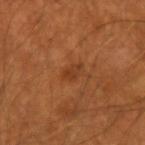Q: Was this lesion biopsied?
A: total-body-photography surveillance lesion; no biopsy
Q: Where on the body is the lesion?
A: the left forearm
Q: How was the tile lit?
A: cross-polarized
Q: What did automated image analysis measure?
A: a border-irregularity rating of about 3/10, internal color variation of about 2.5 on a 0–10 scale, and radial color variation of about 1; a nevus-likeness score of about 5/100 and lesion-presence confidence of about 100/100
Q: What is the lesion's diameter?
A: about 2.5 mm
Q: Patient demographics?
A: male, approximately 50 years of age
Q: What kind of image is this?
A: ~15 mm crop, total-body skin-cancer survey